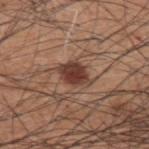Case summary:
• notes · imaged on a skin check; not biopsied
• tile lighting · white-light illumination
• lesion size · ≈4 mm
• image · total-body-photography crop, ~15 mm field of view
• location · the upper back
• patient · male, aged 58 to 62